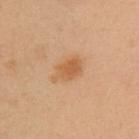Recorded during total-body skin imaging; not selected for excision or biopsy. The patient is a female approximately 50 years of age. The lesion is on the chest. A 15 mm close-up extracted from a 3D total-body photography capture. Imaged with cross-polarized lighting.